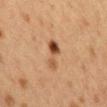Q: Was this lesion biopsied?
A: no biopsy performed (imaged during a skin exam)
Q: How was the tile lit?
A: cross-polarized
Q: What is the imaging modality?
A: total-body-photography crop, ~15 mm field of view
Q: What is the lesion's diameter?
A: ~4 mm (longest diameter)
Q: What are the patient's age and sex?
A: female, about 40 years old
Q: Lesion location?
A: the mid back